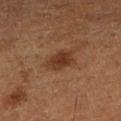Recorded during total-body skin imaging; not selected for excision or biopsy. On the leg. Cropped from a whole-body photographic skin survey; the tile spans about 15 mm. A male subject, aged around 75. The lesion's longest dimension is about 4 mm.